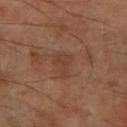Recorded during total-body skin imaging; not selected for excision or biopsy. A close-up tile cropped from a whole-body skin photograph, about 15 mm across. The lesion's longest dimension is about 2.5 mm. The tile uses cross-polarized illumination. A male patient, aged 68–72. The lesion is on the right forearm.